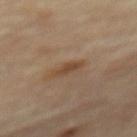| key | value |
|---|---|
| biopsy status | no biopsy performed (imaged during a skin exam) |
| anatomic site | the mid back |
| subject | male, approximately 85 years of age or older |
| lighting | cross-polarized illumination |
| acquisition | 15 mm crop, total-body photography |
| lesion diameter | ≈3 mm |